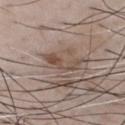biopsy status: catalogued during a skin exam; not biopsied